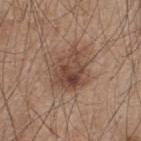- biopsy status: total-body-photography surveillance lesion; no biopsy
- patient: male, aged 43–47
- image: 15 mm crop, total-body photography
- automated lesion analysis: a lesion color around L≈46 a*≈18 b*≈27 in CIELAB and a lesion–skin lightness drop of about 10
- diameter: about 5.5 mm
- location: the upper back
- tile lighting: white-light illumination